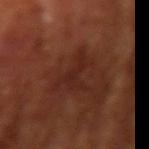The lesion was tiled from a total-body skin photograph and was not biopsied. The recorded lesion diameter is about 4 mm. Cropped from a total-body skin-imaging series; the visible field is about 15 mm. The subject is a male aged 63–67. The tile uses cross-polarized illumination. On the arm.